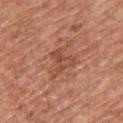Clinical impression: Recorded during total-body skin imaging; not selected for excision or biopsy. Acquisition and patient details: This image is a 15 mm lesion crop taken from a total-body photograph. The patient is a male aged approximately 65. The lesion is on the upper back. Approximately 4 mm at its widest. Imaged with white-light lighting.